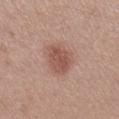A roughly 15 mm field-of-view crop from a total-body skin photograph. This is a white-light tile. The patient is a female about 30 years old. The lesion is located on the right lower leg.